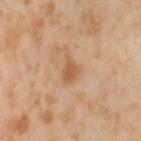tile lighting = cross-polarized illumination | location = the left thigh | acquisition = ~15 mm crop, total-body skin-cancer survey | subject = female, in their mid-50s | lesion diameter = ≈3 mm.